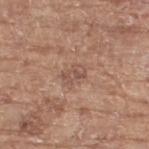– workup: total-body-photography surveillance lesion; no biopsy
– automated lesion analysis: a border-irregularity index near 4/10, a color-variation rating of about 2/10, and a peripheral color-asymmetry measure near 0.5; a nevus-likeness score of about 0/100
– image source: ~15 mm tile from a whole-body skin photo
– anatomic site: the right thigh
– subject: female, aged 73 to 77